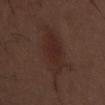Q: What are the patient's age and sex?
A: male, aged around 70
Q: Lesion location?
A: the front of the torso
Q: What is the imaging modality?
A: ~15 mm crop, total-body skin-cancer survey